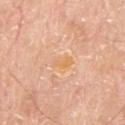Clinical impression:
Captured during whole-body skin photography for melanoma surveillance; the lesion was not biopsied.
Background:
Automated tile analysis of the lesion measured a border-irregularity index near 3/10, internal color variation of about 1 on a 0–10 scale, and peripheral color asymmetry of about 0. And it measured a classifier nevus-likeness of about 0/100 and a lesion-detection confidence of about 100/100. On the upper back. Cropped from a whole-body photographic skin survey; the tile spans about 15 mm. The recorded lesion diameter is about 2.5 mm. Captured under white-light illumination. A male patient in their 80s.